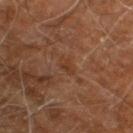- imaging modality: ~15 mm crop, total-body skin-cancer survey
- illumination: cross-polarized
- patient: male, about 60 years old
- location: the right leg